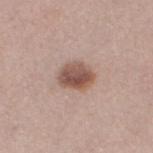workup = total-body-photography surveillance lesion; no biopsy | imaging modality = ~15 mm crop, total-body skin-cancer survey | subject = female, aged 38 to 42 | anatomic site = the left thigh | lighting = white-light illumination.